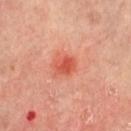This lesion was catalogued during total-body skin photography and was not selected for biopsy. This is a cross-polarized tile. A lesion tile, about 15 mm wide, cut from a 3D total-body photograph. A female patient aged around 65. An algorithmic analysis of the crop reported a lesion color around L≈55 a*≈36 b*≈36 in CIELAB, a lesion–skin lightness drop of about 10, and a normalized border contrast of about 7.5. It also reported a border-irregularity rating of about 1.5/10, a color-variation rating of about 3.5/10, and a peripheral color-asymmetry measure near 1. And it measured an automated nevus-likeness rating near 60 out of 100 and lesion-presence confidence of about 100/100. The lesion is located on the right leg. Measured at roughly 3 mm in maximum diameter.